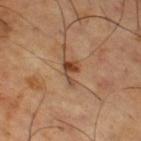The lesion was photographed on a routine skin check and not biopsied; there is no pathology result. A male patient aged approximately 65. The lesion is on the right thigh. Automated tile analysis of the lesion measured a footprint of about 3.5 mm², an outline eccentricity of about 0.8 (0 = round, 1 = elongated), and a shape-asymmetry score of about 0.55 (0 = symmetric). It also reported a border-irregularity rating of about 6.5/10, internal color variation of about 2.5 on a 0–10 scale, and peripheral color asymmetry of about 1. And it measured an automated nevus-likeness rating near 45 out of 100. A region of skin cropped from a whole-body photographic capture, roughly 15 mm wide.